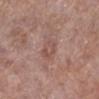Q: Was a biopsy performed?
A: total-body-photography surveillance lesion; no biopsy
Q: What is the lesion's diameter?
A: about 2.5 mm
Q: What lighting was used for the tile?
A: white-light
Q: Lesion location?
A: the left lower leg
Q: What did automated image analysis measure?
A: an area of roughly 4 mm², an outline eccentricity of about 0.75 (0 = round, 1 = elongated), and a shape-asymmetry score of about 0.3 (0 = symmetric); border irregularity of about 2.5 on a 0–10 scale and a peripheral color-asymmetry measure near 1
Q: What are the patient's age and sex?
A: female, about 75 years old
Q: What kind of image is this?
A: total-body-photography crop, ~15 mm field of view